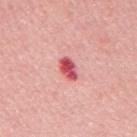Context:
Approximately 3 mm at its widest. A male subject, approximately 50 years of age. The lesion is located on the mid back. A 15 mm close-up tile from a total-body photography series done for melanoma screening. The tile uses white-light illumination.